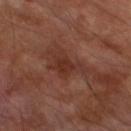Captured during whole-body skin photography for melanoma surveillance; the lesion was not biopsied. This image is a 15 mm lesion crop taken from a total-body photograph. From the left lower leg. An algorithmic analysis of the crop reported border irregularity of about 5.5 on a 0–10 scale, a within-lesion color-variation index near 2.5/10, and peripheral color asymmetry of about 0.5. Captured under cross-polarized illumination. The recorded lesion diameter is about 4 mm. A male patient roughly 70 years of age.